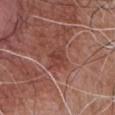biopsy status=imaged on a skin check; not biopsied | subject=male, about 75 years old | site=the front of the torso | acquisition=15 mm crop, total-body photography | diameter=about 2.5 mm | illumination=white-light illumination.